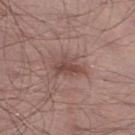Recorded during total-body skin imaging; not selected for excision or biopsy.
Captured under white-light illumination.
A male patient, approximately 55 years of age.
On the right lower leg.
Approximately 3.5 mm at its widest.
Cropped from a total-body skin-imaging series; the visible field is about 15 mm.